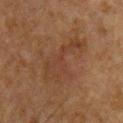follow-up: total-body-photography surveillance lesion; no biopsy | anatomic site: the front of the torso | acquisition: ~15 mm crop, total-body skin-cancer survey | subject: male, about 50 years old.